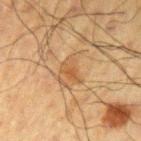follow-up = total-body-photography surveillance lesion; no biopsy
image source = 15 mm crop, total-body photography
subject = male, in their 60s
body site = the left upper arm